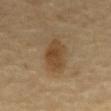Recorded during total-body skin imaging; not selected for excision or biopsy.
Cropped from a total-body skin-imaging series; the visible field is about 15 mm.
The total-body-photography lesion software estimated a shape eccentricity near 0.85 and two-axis asymmetry of about 0.15. And it measured a border-irregularity index near 2/10, internal color variation of about 3.5 on a 0–10 scale, and a peripheral color-asymmetry measure near 1. The software also gave a nevus-likeness score of about 100/100 and a lesion-detection confidence of about 100/100.
The recorded lesion diameter is about 5 mm.
A male patient, roughly 65 years of age.
Imaged with cross-polarized lighting.
From the mid back.